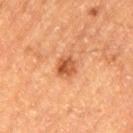Notes:
• notes — no biopsy performed (imaged during a skin exam)
• patient — male, aged 83 or older
• acquisition — ~15 mm crop, total-body skin-cancer survey
• anatomic site — the left thigh